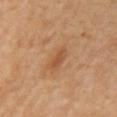{
  "biopsy_status": "not biopsied; imaged during a skin examination",
  "lesion_size": {
    "long_diameter_mm_approx": 2.5
  },
  "automated_metrics": {
    "area_mm2_approx": 3.5,
    "shape_asymmetry": 0.25,
    "nevus_likeness_0_100": 35,
    "lesion_detection_confidence_0_100": 100
  },
  "image": {
    "source": "total-body photography crop",
    "field_of_view_mm": 15
  },
  "patient": {
    "sex": "male",
    "age_approx": 65
  },
  "site": "mid back",
  "lighting": "cross-polarized"
}